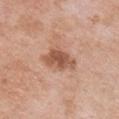A female patient, aged 48 to 52.
Cropped from a whole-body photographic skin survey; the tile spans about 15 mm.
This is a white-light tile.
From the chest.
Measured at roughly 4.5 mm in maximum diameter.
An algorithmic analysis of the crop reported a lesion area of about 9 mm², an eccentricity of roughly 0.75, and a shape-asymmetry score of about 0.3 (0 = symmetric). It also reported a mean CIELAB color near L≈55 a*≈23 b*≈30 and about 12 CIELAB-L* units darker than the surrounding skin. The analysis additionally found border irregularity of about 3.5 on a 0–10 scale, a within-lesion color-variation index near 3.5/10, and peripheral color asymmetry of about 1. The software also gave an automated nevus-likeness rating near 45 out of 100 and lesion-presence confidence of about 100/100.